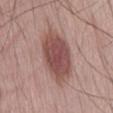Clinical impression:
The lesion was tiled from a total-body skin photograph and was not biopsied.
Background:
A 15 mm close-up tile from a total-body photography series done for melanoma screening. The lesion is on the abdomen. A male subject, aged around 70. Imaged with white-light lighting.